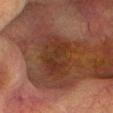{"biopsy_status": "not biopsied; imaged during a skin examination", "lighting": "cross-polarized", "image": {"source": "total-body photography crop", "field_of_view_mm": 15}, "lesion_size": {"long_diameter_mm_approx": 5.5}, "automated_metrics": {"area_mm2_approx": 18.0, "eccentricity": 0.75, "shape_asymmetry": 0.2, "vs_skin_darker_L": 6.0, "nevus_likeness_0_100": 0}, "site": "head or neck", "patient": {"sex": "male", "age_approx": 70}}